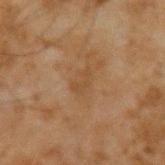Measured at roughly 2.5 mm in maximum diameter. A roughly 15 mm field-of-view crop from a total-body skin photograph. A male patient, approximately 45 years of age. From the arm.